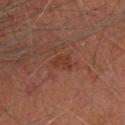notes = no biopsy performed (imaged during a skin exam)